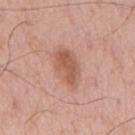  biopsy_status: not biopsied; imaged during a skin examination
  patient:
    sex: male
    age_approx: 65
  lighting: white-light
  image:
    source: total-body photography crop
    field_of_view_mm: 15
  lesion_size:
    long_diameter_mm_approx: 5.0
  site: back
  automated_metrics:
    color_variation_0_10: 4.0
    peripheral_color_asymmetry: 1.5